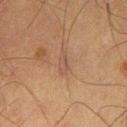The lesion was tiled from a total-body skin photograph and was not biopsied. An algorithmic analysis of the crop reported a lesion area of about 3.5 mm², a shape eccentricity near 0.85, and a shape-asymmetry score of about 0.2 (0 = symmetric). On the leg. The patient is a male in their mid- to late 60s. A 15 mm close-up tile from a total-body photography series done for melanoma screening. Measured at roughly 2.5 mm in maximum diameter.